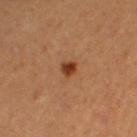The lesion was tiled from a total-body skin photograph and was not biopsied. This image is a 15 mm lesion crop taken from a total-body photograph. A female patient in their mid-50s. The tile uses cross-polarized illumination. From the left thigh. Longest diameter approximately 2 mm. The lesion-visualizer software estimated a border-irregularity index near 2.5/10.